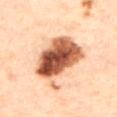Context:
A female subject aged around 60. Approximately 8 mm at its widest. Imaged with cross-polarized lighting. A roughly 15 mm field-of-view crop from a total-body skin photograph. The lesion is on the back.